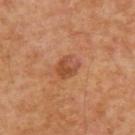<case>
<biopsy_status>not biopsied; imaged during a skin examination</biopsy_status>
<image>
  <source>total-body photography crop</source>
  <field_of_view_mm>15</field_of_view_mm>
</image>
<lighting>cross-polarized</lighting>
<site>upper back</site>
<patient>
  <sex>male</sex>
  <age_approx>55</age_approx>
</patient>
<lesion_size>
  <long_diameter_mm_approx>3.0</long_diameter_mm_approx>
</lesion_size>
</case>